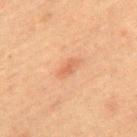Q: Was a biopsy performed?
A: total-body-photography surveillance lesion; no biopsy
Q: Illumination type?
A: cross-polarized illumination
Q: What is the imaging modality?
A: total-body-photography crop, ~15 mm field of view
Q: Lesion size?
A: ~2.5 mm (longest diameter)
Q: Lesion location?
A: the upper back
Q: What are the patient's age and sex?
A: male, aged around 60